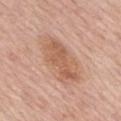Recorded during total-body skin imaging; not selected for excision or biopsy. This is a white-light tile. A lesion tile, about 15 mm wide, cut from a 3D total-body photograph. Longest diameter approximately 6.5 mm. From the mid back. A female patient aged 63 to 67.